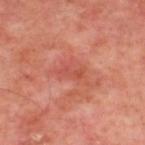{"biopsy_status": "not biopsied; imaged during a skin examination", "image": {"source": "total-body photography crop", "field_of_view_mm": 15}, "patient": {"age_approx": 55}, "lesion_size": {"long_diameter_mm_approx": 3.0}, "lighting": "cross-polarized", "site": "upper back"}